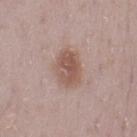Recorded during total-body skin imaging; not selected for excision or biopsy.
A region of skin cropped from a whole-body photographic capture, roughly 15 mm wide.
Captured under white-light illumination.
The subject is a female aged approximately 30.
The lesion's longest dimension is about 4.5 mm.
Located on the left thigh.